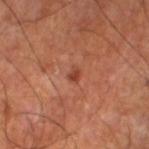Notes:
– workup: imaged on a skin check; not biopsied
– anatomic site: the left lower leg
– image source: total-body-photography crop, ~15 mm field of view
– diameter: ≈1.5 mm
– image-analysis metrics: an area of roughly 2 mm² and a shape-asymmetry score of about 0.3 (0 = symmetric); border irregularity of about 2.5 on a 0–10 scale, internal color variation of about 0 on a 0–10 scale, and radial color variation of about 0; a classifier nevus-likeness of about 65/100 and a detector confidence of about 100 out of 100 that the crop contains a lesion
– lighting: cross-polarized illumination
– subject: male, roughly 60 years of age